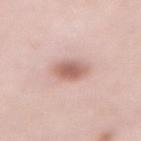automated lesion analysis — a shape eccentricity near 0.55 and a symmetry-axis asymmetry near 0.15; border irregularity of about 1.5 on a 0–10 scale, a within-lesion color-variation index near 3.5/10, and peripheral color asymmetry of about 1; an automated nevus-likeness rating near 95 out of 100
site — the abdomen
lesion size — about 3 mm
subject — female, in their 50s
illumination — white-light
acquisition — 15 mm crop, total-body photography